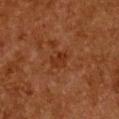Q: What kind of image is this?
A: ~15 mm tile from a whole-body skin photo
Q: What is the anatomic site?
A: the chest
Q: Patient demographics?
A: female, approximately 50 years of age
Q: How was the tile lit?
A: cross-polarized